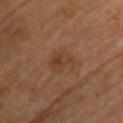- image source: ~15 mm crop, total-body skin-cancer survey
- body site: the front of the torso
- patient: female